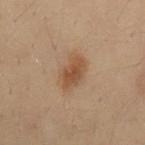Q: Is there a histopathology result?
A: catalogued during a skin exam; not biopsied
Q: What kind of image is this?
A: 15 mm crop, total-body photography
Q: Who is the patient?
A: male, aged 28–32
Q: What is the anatomic site?
A: the mid back